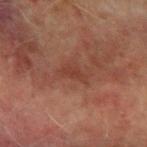{"biopsy_status": "not biopsied; imaged during a skin examination", "lesion_size": {"long_diameter_mm_approx": 3.0}, "automated_metrics": {"area_mm2_approx": 3.0, "eccentricity": 0.9, "shape_asymmetry": 0.4, "border_irregularity_0_10": 4.5, "color_variation_0_10": 0.5}, "patient": {"sex": "male", "age_approx": 70}, "lighting": "cross-polarized", "image": {"source": "total-body photography crop", "field_of_view_mm": 15}, "site": "left forearm"}